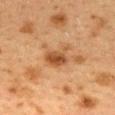Case summary:
• follow-up: no biopsy performed (imaged during a skin exam)
• anatomic site: the mid back
• imaging modality: ~15 mm tile from a whole-body skin photo
• lesion diameter: ~3.5 mm (longest diameter)
• illumination: cross-polarized illumination
• patient: female, aged 38 to 42
• image-analysis metrics: a lesion area of about 6 mm², an eccentricity of roughly 0.8, and two-axis asymmetry of about 0.35; a lesion color around L≈42 a*≈20 b*≈34 in CIELAB, about 10 CIELAB-L* units darker than the surrounding skin, and a normalized lesion–skin contrast near 8.5; a classifier nevus-likeness of about 80/100 and a detector confidence of about 100 out of 100 that the crop contains a lesion